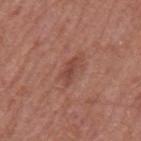Captured during whole-body skin photography for melanoma surveillance; the lesion was not biopsied.
Cropped from a total-body skin-imaging series; the visible field is about 15 mm.
The tile uses white-light illumination.
The lesion is on the mid back.
Approximately 3.5 mm at its widest.
Automated tile analysis of the lesion measured a lesion area of about 4 mm², a shape eccentricity near 0.9, and a symmetry-axis asymmetry near 0.35. It also reported a mean CIELAB color near L≈45 a*≈25 b*≈26 and about 8 CIELAB-L* units darker than the surrounding skin. The software also gave a border-irregularity index near 4/10, a within-lesion color-variation index near 3/10, and radial color variation of about 1.5. The analysis additionally found a detector confidence of about 100 out of 100 that the crop contains a lesion.
The patient is a male roughly 65 years of age.